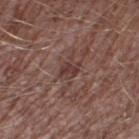• biopsy status — catalogued during a skin exam; not biopsied
• site — the right thigh
• patient — male, roughly 45 years of age
• automated lesion analysis — a footprint of about 4 mm², an eccentricity of roughly 0.65, and a symmetry-axis asymmetry near 0.2; a lesion–skin lightness drop of about 8; border irregularity of about 2.5 on a 0–10 scale, a within-lesion color-variation index near 3/10, and radial color variation of about 1
• acquisition — ~15 mm crop, total-body skin-cancer survey
• illumination — white-light illumination